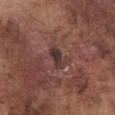Notes:
- biopsy status · total-body-photography surveillance lesion; no biopsy
- site · the abdomen
- acquisition · total-body-photography crop, ~15 mm field of view
- lesion diameter · ≈3 mm
- patient · male, aged 73–77
- tile lighting · white-light illumination